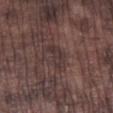notes: imaged on a skin check; not biopsied | body site: the right lower leg | tile lighting: white-light | acquisition: 15 mm crop, total-body photography | patient: male, aged approximately 75 | lesion diameter: ~3.5 mm (longest diameter).